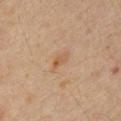{"biopsy_status": "not biopsied; imaged during a skin examination", "site": "abdomen", "lighting": "cross-polarized", "patient": {"sex": "male", "age_approx": 60}, "image": {"source": "total-body photography crop", "field_of_view_mm": 15}, "automated_metrics": {"area_mm2_approx": 3.0, "eccentricity": 0.85, "shape_asymmetry": 0.25, "color_variation_0_10": 1.5, "peripheral_color_asymmetry": 0.5}}